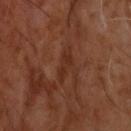The lesion was tiled from a total-body skin photograph and was not biopsied.
A lesion tile, about 15 mm wide, cut from a 3D total-body photograph.
Captured under cross-polarized illumination.
The lesion is on the head or neck.
Longest diameter approximately 4 mm.
A male patient, in their 60s.
An algorithmic analysis of the crop reported a lesion area of about 4.5 mm², a shape eccentricity near 0.95, and a shape-asymmetry score of about 0.25 (0 = symmetric). The software also gave border irregularity of about 4 on a 0–10 scale.